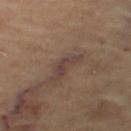Findings:
- workup: total-body-photography surveillance lesion; no biopsy
- location: the left thigh
- diameter: about 3.5 mm
- automated lesion analysis: a color-variation rating of about 0/10 and peripheral color asymmetry of about 0
- patient: aged 58–62
- acquisition: 15 mm crop, total-body photography